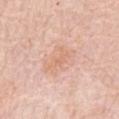{
  "image": {
    "source": "total-body photography crop",
    "field_of_view_mm": 15
  },
  "lesion_size": {
    "long_diameter_mm_approx": 3.5
  },
  "site": "abdomen",
  "patient": {
    "sex": "male",
    "age_approx": 80
  },
  "automated_metrics": {
    "area_mm2_approx": 4.5,
    "eccentricity": 0.9,
    "shape_asymmetry": 0.4,
    "peripheral_color_asymmetry": 0.0,
    "nevus_likeness_0_100": 0
  }
}